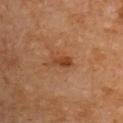No biopsy was performed on this lesion — it was imaged during a full skin examination and was not determined to be concerning. Imaged with cross-polarized lighting. The lesion is on the right upper arm. A male subject, in their mid- to late 50s. Longest diameter approximately 2.5 mm. A 15 mm close-up tile from a total-body photography series done for melanoma screening.